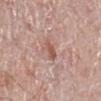  biopsy_status: not biopsied; imaged during a skin examination
  image:
    source: total-body photography crop
    field_of_view_mm: 15
  patient:
    sex: male
    age_approx: 70
  lighting: white-light
  lesion_size:
    long_diameter_mm_approx: 2.5
  site: right leg